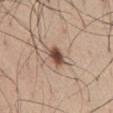Recorded during total-body skin imaging; not selected for excision or biopsy.
A male subject, aged 58 to 62.
The tile uses white-light illumination.
On the abdomen.
An algorithmic analysis of the crop reported a border-irregularity rating of about 2/10, a within-lesion color-variation index near 4/10, and peripheral color asymmetry of about 1.
The recorded lesion diameter is about 3 mm.
A 15 mm crop from a total-body photograph taken for skin-cancer surveillance.